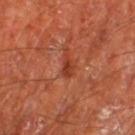{"biopsy_status": "not biopsied; imaged during a skin examination", "lighting": "cross-polarized", "image": {"source": "total-body photography crop", "field_of_view_mm": 15}, "lesion_size": {"long_diameter_mm_approx": 3.0}, "patient": {"sex": "male", "age_approx": 65}, "site": "left thigh"}